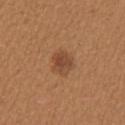Imaged during a routine full-body skin examination; the lesion was not biopsied and no histopathology is available. From the left upper arm. The subject is a female aged 38–42. Longest diameter approximately 3 mm. Imaged with white-light lighting. A 15 mm close-up extracted from a 3D total-body photography capture.